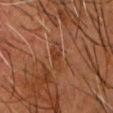biopsy status: no biopsy performed (imaged during a skin exam) | image: 15 mm crop, total-body photography | subject: male, approximately 60 years of age | lesion diameter: ~4.5 mm (longest diameter) | lighting: cross-polarized | body site: the head or neck | automated lesion analysis: border irregularity of about 4 on a 0–10 scale, a within-lesion color-variation index near 3.5/10, and peripheral color asymmetry of about 1; a classifier nevus-likeness of about 0/100 and a lesion-detection confidence of about 80/100.